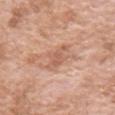Case summary:
* notes: no biopsy performed (imaged during a skin exam)